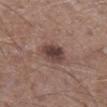biopsy status: total-body-photography surveillance lesion; no biopsy | location: the left lower leg | subject: male, about 45 years old | illumination: white-light | size: ~3 mm (longest diameter) | TBP lesion metrics: a footprint of about 7.5 mm² and an outline eccentricity of about 0.15 (0 = round, 1 = elongated); border irregularity of about 1.5 on a 0–10 scale and radial color variation of about 1.5 | image: 15 mm crop, total-body photography.